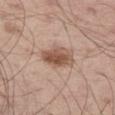{
  "biopsy_status": "not biopsied; imaged during a skin examination",
  "site": "leg",
  "lighting": "white-light",
  "lesion_size": {
    "long_diameter_mm_approx": 4.0
  },
  "patient": {
    "sex": "male",
    "age_approx": 55
  },
  "image": {
    "source": "total-body photography crop",
    "field_of_view_mm": 15
  }
}